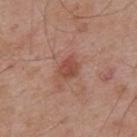biopsy status = total-body-photography surveillance lesion; no biopsy
image = ~15 mm crop, total-body skin-cancer survey
site = the mid back
lesion diameter = about 2.5 mm
subject = male, aged around 55